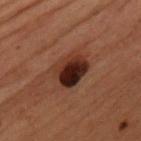Impression:
Part of a total-body skin-imaging series; this lesion was reviewed on a skin check and was not flagged for biopsy.
Clinical summary:
This image is a 15 mm lesion crop taken from a total-body photograph. The subject is a male aged 48–52. The tile uses cross-polarized illumination. Approximately 4.5 mm at its widest. On the chest.